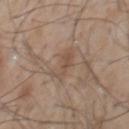From the front of the torso. Automated image analysis of the tile measured about 7 CIELAB-L* units darker than the surrounding skin. A 15 mm close-up extracted from a 3D total-body photography capture. Captured under white-light illumination. A male subject, aged around 60. About 3.5 mm across.